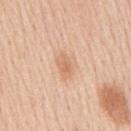Clinical impression:
Imaged during a routine full-body skin examination; the lesion was not biopsied and no histopathology is available.